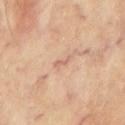follow-up: imaged on a skin check; not biopsied | automated metrics: an eccentricity of roughly 0.95 and a shape-asymmetry score of about 0.5 (0 = symmetric); a mean CIELAB color near L≈63 a*≈21 b*≈29, a lesion–skin lightness drop of about 8, and a lesion-to-skin contrast of about 5 (normalized; higher = more distinct); a border-irregularity index near 5/10 and a peripheral color-asymmetry measure near 0 | diameter: ~2.5 mm (longest diameter) | patient: male, in their 70s | imaging modality: 15 mm crop, total-body photography | site: the chest | tile lighting: cross-polarized illumination.